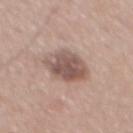Assessment:
Recorded during total-body skin imaging; not selected for excision or biopsy.